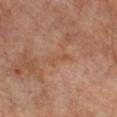{"biopsy_status": "not biopsied; imaged during a skin examination", "patient": {"sex": "male", "age_approx": 70}, "site": "chest", "automated_metrics": {"area_mm2_approx": 2.0, "eccentricity": 0.95, "shape_asymmetry": 0.6, "cielab_L": 49, "cielab_a": 21, "cielab_b": 32, "vs_skin_darker_L": 5.0}, "image": {"source": "total-body photography crop", "field_of_view_mm": 15}, "lighting": "cross-polarized", "lesion_size": {"long_diameter_mm_approx": 3.0}}